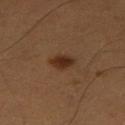Notes:
– biopsy status — total-body-photography surveillance lesion; no biopsy
– location — the left thigh
– acquisition — 15 mm crop, total-body photography
– subject — male, approximately 55 years of age
– tile lighting — cross-polarized illumination
– automated metrics — a border-irregularity rating of about 2/10, a color-variation rating of about 3/10, and radial color variation of about 1; an automated nevus-likeness rating near 100 out of 100 and lesion-presence confidence of about 100/100
– lesion size — about 3 mm